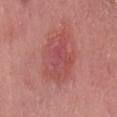Findings:
– biopsy status · total-body-photography surveillance lesion; no biopsy
– anatomic site · the lower back
– subject · male, in their 40s
– acquisition · ~15 mm tile from a whole-body skin photo
– lesion diameter · about 6.5 mm
– automated lesion analysis · a footprint of about 20 mm², an eccentricity of roughly 0.75, and two-axis asymmetry of about 0.2; a lesion color around L≈51 a*≈30 b*≈24 in CIELAB, about 8 CIELAB-L* units darker than the surrounding skin, and a normalized lesion–skin contrast near 5.5; border irregularity of about 2.5 on a 0–10 scale and peripheral color asymmetry of about 1.5; a classifier nevus-likeness of about 5/100 and lesion-presence confidence of about 100/100
– illumination · white-light illumination